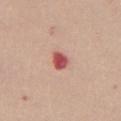This lesion was catalogued during total-body skin photography and was not selected for biopsy. Located on the abdomen. A female subject, aged 38–42. A lesion tile, about 15 mm wide, cut from a 3D total-body photograph.